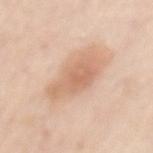Part of a total-body skin-imaging series; this lesion was reviewed on a skin check and was not flagged for biopsy.
The lesion-visualizer software estimated an area of roughly 16 mm², a shape eccentricity near 0.8, and two-axis asymmetry of about 0.25. The analysis additionally found a lesion color around L≈67 a*≈20 b*≈32 in CIELAB, about 10 CIELAB-L* units darker than the surrounding skin, and a lesion-to-skin contrast of about 6 (normalized; higher = more distinct). The software also gave internal color variation of about 3 on a 0–10 scale and a peripheral color-asymmetry measure near 1. The software also gave a lesion-detection confidence of about 100/100.
A female patient, roughly 65 years of age.
This is a white-light tile.
From the mid back.
Approximately 6 mm at its widest.
A region of skin cropped from a whole-body photographic capture, roughly 15 mm wide.